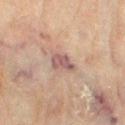<lesion>
<biopsy_status>not biopsied; imaged during a skin examination</biopsy_status>
<lighting>cross-polarized</lighting>
<lesion_size>
  <long_diameter_mm_approx>3.0</long_diameter_mm_approx>
</lesion_size>
<site>right thigh</site>
<image>
  <source>total-body photography crop</source>
  <field_of_view_mm>15</field_of_view_mm>
</image>
<patient>
  <sex>female</sex>
  <age_approx>80</age_approx>
</patient>
</lesion>